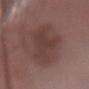No biopsy was performed on this lesion — it was imaged during a full skin examination and was not determined to be concerning. On the arm. The tile uses white-light illumination. An algorithmic analysis of the crop reported an area of roughly 23 mm², an outline eccentricity of about 0.7 (0 = round, 1 = elongated), and a symmetry-axis asymmetry near 0.3. It also reported border irregularity of about 3.5 on a 0–10 scale and a color-variation rating of about 2.5/10. About 6.5 mm across. The subject is a male aged 73 to 77. A 15 mm close-up extracted from a 3D total-body photography capture.